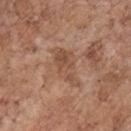  biopsy_status: not biopsied; imaged during a skin examination
  lighting: white-light
  site: chest
  patient:
    sex: male
    age_approx: 70
  lesion_size:
    long_diameter_mm_approx: 5.0
  image:
    source: total-body photography crop
    field_of_view_mm: 15
  automated_metrics:
    cielab_L: 50
    cielab_a: 20
    cielab_b: 30
    vs_skin_contrast_norm: 6.0
    border_irregularity_0_10: 5.0
    nevus_likeness_0_100: 0